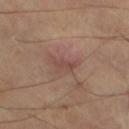workup: catalogued during a skin exam; not biopsied
location: the right leg
imaging modality: ~15 mm crop, total-body skin-cancer survey
subject: female, in their 60s
illumination: cross-polarized illumination